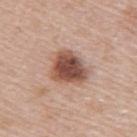Assessment: Captured during whole-body skin photography for melanoma surveillance; the lesion was not biopsied. Image and clinical context: The lesion is on the right upper arm. A female subject, aged approximately 50. This is a white-light tile. This image is a 15 mm lesion crop taken from a total-body photograph.